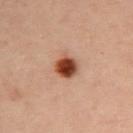Recorded during total-body skin imaging; not selected for excision or biopsy. A female patient, about 40 years old. Automated tile analysis of the lesion measured a lesion color around L≈45 a*≈26 b*≈32 in CIELAB and a normalized border contrast of about 13. And it measured border irregularity of about 1.5 on a 0–10 scale. The lesion is on the upper back. The tile uses cross-polarized illumination. Cropped from a total-body skin-imaging series; the visible field is about 15 mm. The recorded lesion diameter is about 3 mm.